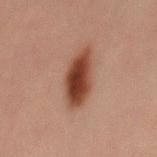Q: Was this lesion biopsied?
A: no biopsy performed (imaged during a skin exam)
Q: What are the patient's age and sex?
A: male, aged approximately 30
Q: What kind of image is this?
A: ~15 mm crop, total-body skin-cancer survey
Q: Where on the body is the lesion?
A: the mid back